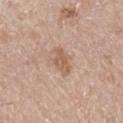workup: imaged on a skin check; not biopsied
imaging modality: total-body-photography crop, ~15 mm field of view
anatomic site: the right lower leg
lighting: white-light
subject: female, aged around 55
diameter: about 3.5 mm